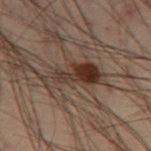biopsy status: no biopsy performed (imaged during a skin exam); subject: male, aged 53 to 57; diameter: ≈6.5 mm; lighting: cross-polarized; location: the left thigh; image: 15 mm crop, total-body photography.